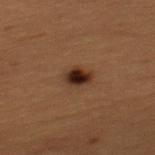Part of a total-body skin-imaging series; this lesion was reviewed on a skin check and was not flagged for biopsy.
Located on the mid back.
A male subject aged 48 to 52.
A close-up tile cropped from a whole-body skin photograph, about 15 mm across.